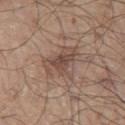Impression:
The lesion was tiled from a total-body skin photograph and was not biopsied.
Image and clinical context:
This is a white-light tile. Cropped from a total-body skin-imaging series; the visible field is about 15 mm. Located on the left thigh. The subject is a male aged around 75.